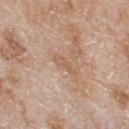The lesion was tiled from a total-body skin photograph and was not biopsied. This image is a 15 mm lesion crop taken from a total-body photograph. The lesion is on the upper back. A male subject about 80 years old. The tile uses white-light illumination.